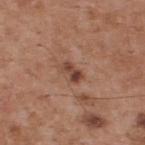No biopsy was performed on this lesion — it was imaged during a full skin examination and was not determined to be concerning. A male patient in their mid-50s. Imaged with white-light lighting. Longest diameter approximately 2.5 mm. A close-up tile cropped from a whole-body skin photograph, about 15 mm across. The lesion is located on the upper back. Automated tile analysis of the lesion measured a lesion area of about 3.5 mm², a shape eccentricity near 0.85, and a symmetry-axis asymmetry near 0.3. The analysis additionally found an average lesion color of about L≈43 a*≈22 b*≈27 (CIELAB), about 12 CIELAB-L* units darker than the surrounding skin, and a normalized border contrast of about 9. The software also gave a color-variation rating of about 2/10 and peripheral color asymmetry of about 0.